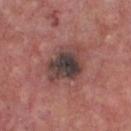The lesion was photographed on a routine skin check and not biopsied; there is no pathology result. A region of skin cropped from a whole-body photographic capture, roughly 15 mm wide. Approximately 4.5 mm at its widest. From the chest. A male subject, approximately 75 years of age. This is a white-light tile.